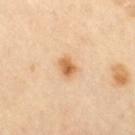notes=imaged on a skin check; not biopsied | subject=male, in their mid-60s | image-analysis metrics=an area of roughly 3.5 mm² and an eccentricity of roughly 0.6; a classifier nevus-likeness of about 100/100 and lesion-presence confidence of about 100/100 | tile lighting=cross-polarized illumination | imaging modality=total-body-photography crop, ~15 mm field of view | site=the leg.